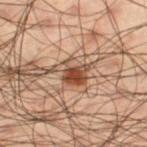workup: catalogued during a skin exam; not biopsied | tile lighting: cross-polarized illumination | patient: male, aged around 50 | site: the right thigh | acquisition: ~15 mm crop, total-body skin-cancer survey.